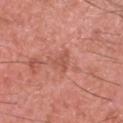Imaged during a routine full-body skin examination; the lesion was not biopsied and no histopathology is available.
A roughly 15 mm field-of-view crop from a total-body skin photograph.
On the head or neck.
A male subject about 45 years old.